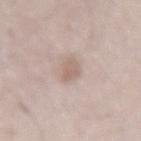location = the lower back | image source = 15 mm crop, total-body photography | subject = male, roughly 50 years of age | lesion diameter = ~3 mm (longest diameter) | illumination = white-light illumination.